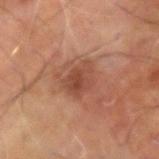Impression: Part of a total-body skin-imaging series; this lesion was reviewed on a skin check and was not flagged for biopsy. Acquisition and patient details: A male subject, aged 58–62. The lesion is located on the right arm. A roughly 15 mm field-of-view crop from a total-body skin photograph.